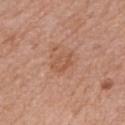Part of a total-body skin-imaging series; this lesion was reviewed on a skin check and was not flagged for biopsy.
The subject is a female aged around 60.
The recorded lesion diameter is about 3 mm.
This image is a 15 mm lesion crop taken from a total-body photograph.
Imaged with white-light lighting.
On the right forearm.